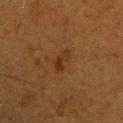biopsy status = catalogued during a skin exam; not biopsied
lighting = cross-polarized
subject = female, aged approximately 40
size = about 3 mm
site = the upper back
image = 15 mm crop, total-body photography